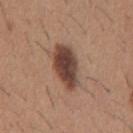lesion size — ≈6 mm | anatomic site — the mid back | image — 15 mm crop, total-body photography | TBP lesion metrics — a normalized border contrast of about 11; a border-irregularity rating of about 2.5/10, a color-variation rating of about 6/10, and a peripheral color-asymmetry measure near 1.5; an automated nevus-likeness rating near 95 out of 100 | lighting — white-light | subject — male, aged around 60.